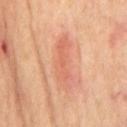No biopsy was performed on this lesion — it was imaged during a full skin examination and was not determined to be concerning.
Automated image analysis of the tile measured a lesion area of about 11 mm² and a symmetry-axis asymmetry near 0.35. The software also gave an average lesion color of about L≈61 a*≈26 b*≈33 (CIELAB), roughly 8 lightness units darker than nearby skin, and a normalized lesion–skin contrast near 5. The analysis additionally found a nevus-likeness score of about 10/100.
The lesion's longest dimension is about 6.5 mm.
From the front of the torso.
The tile uses cross-polarized illumination.
A male subject approximately 70 years of age.
A close-up tile cropped from a whole-body skin photograph, about 15 mm across.